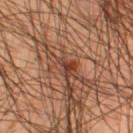The lesion was tiled from a total-body skin photograph and was not biopsied.
A male subject, aged around 45.
The recorded lesion diameter is about 6 mm.
A roughly 15 mm field-of-view crop from a total-body skin photograph.
The lesion is on the right thigh.